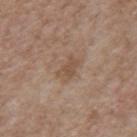Imaged during a routine full-body skin examination; the lesion was not biopsied and no histopathology is available. A male patient in their 70s. The total-body-photography lesion software estimated an area of roughly 4.5 mm², a shape eccentricity near 0.8, and two-axis asymmetry of about 0.25. The software also gave an average lesion color of about L≈50 a*≈17 b*≈28 (CIELAB), about 7 CIELAB-L* units darker than the surrounding skin, and a normalized border contrast of about 6. And it measured a classifier nevus-likeness of about 0/100 and a lesion-detection confidence of about 100/100. Captured under white-light illumination. Cropped from a whole-body photographic skin survey; the tile spans about 15 mm. On the mid back.